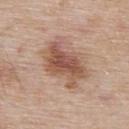biopsy status — no biopsy performed (imaged during a skin exam)
lighting — white-light illumination
lesion diameter — about 5.5 mm
site — the upper back
acquisition — 15 mm crop, total-body photography
automated lesion analysis — a lesion area of about 16 mm², an outline eccentricity of about 0.75 (0 = round, 1 = elongated), and a shape-asymmetry score of about 0.3 (0 = symmetric); a lesion color around L≈53 a*≈21 b*≈29 in CIELAB and a lesion–skin lightness drop of about 12; a border-irregularity rating of about 4.5/10 and internal color variation of about 4.5 on a 0–10 scale
subject — male, in their mid- to late 50s